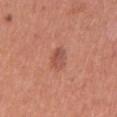The tile uses white-light illumination. A female subject, approximately 30 years of age. Automated tile analysis of the lesion measured a footprint of about 4.5 mm², an outline eccentricity of about 0.8 (0 = round, 1 = elongated), and two-axis asymmetry of about 0.3. It also reported border irregularity of about 3 on a 0–10 scale. It also reported lesion-presence confidence of about 100/100. Approximately 3 mm at its widest. A 15 mm crop from a total-body photograph taken for skin-cancer surveillance. From the left upper arm.